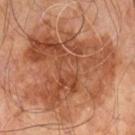{"biopsy_status": "not biopsied; imaged during a skin examination", "site": "right leg", "patient": {"sex": "male", "age_approx": 60}, "lighting": "cross-polarized", "image": {"source": "total-body photography crop", "field_of_view_mm": 15}, "lesion_size": {"long_diameter_mm_approx": 11.0}}